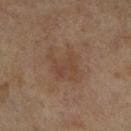Clinical impression: Imaged during a routine full-body skin examination; the lesion was not biopsied and no histopathology is available. Background: The lesion is located on the leg. A 15 mm crop from a total-body photograph taken for skin-cancer surveillance. Imaged with cross-polarized lighting. A female patient, in their mid-60s. The lesion's longest dimension is about 5 mm.